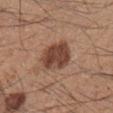Notes:
- image — ~15 mm tile from a whole-body skin photo
- location — the right lower leg
- size — about 4.5 mm
- TBP lesion metrics — a lesion area of about 12 mm² and a shape-asymmetry score of about 0.15 (0 = symmetric); a normalized lesion–skin contrast near 10; a color-variation rating of about 4/10 and radial color variation of about 1.5; an automated nevus-likeness rating near 85 out of 100 and a detector confidence of about 100 out of 100 that the crop contains a lesion
- illumination — white-light
- patient — male, approximately 35 years of age